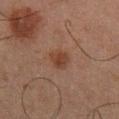The lesion was photographed on a routine skin check and not biopsied; there is no pathology result. Cropped from a whole-body photographic skin survey; the tile spans about 15 mm. A male subject aged around 65. The total-body-photography lesion software estimated a lesion area of about 4.5 mm², a shape eccentricity near 0.55, and a shape-asymmetry score of about 0.3 (0 = symmetric). The software also gave a classifier nevus-likeness of about 85/100. Located on the right lower leg. Approximately 2.5 mm at its widest.